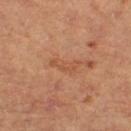Notes:
• follow-up — no biopsy performed (imaged during a skin exam)
• lighting — cross-polarized illumination
• body site — the right thigh
• size — about 3.5 mm
• patient — female, about 60 years old
• image — ~15 mm crop, total-body skin-cancer survey